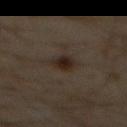Case summary:
– follow-up: imaged on a skin check; not biopsied
– subject: male, aged approximately 60
– acquisition: ~15 mm crop, total-body skin-cancer survey
– body site: the abdomen